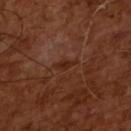Impression: No biopsy was performed on this lesion — it was imaged during a full skin examination and was not determined to be concerning. Acquisition and patient details: About 2.5 mm across. Cropped from a total-body skin-imaging series; the visible field is about 15 mm. A male subject, aged 63–67. This is a cross-polarized tile. The lesion-visualizer software estimated a lesion color around L≈26 a*≈22 b*≈27 in CIELAB, about 5 CIELAB-L* units darker than the surrounding skin, and a normalized lesion–skin contrast near 6.5.